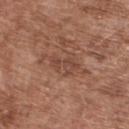follow-up: imaged on a skin check; not biopsied
subject: male, aged 73 to 77
acquisition: total-body-photography crop, ~15 mm field of view
illumination: white-light
anatomic site: the upper back
diameter: about 4 mm